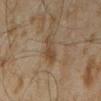Assessment: This lesion was catalogued during total-body skin photography and was not selected for biopsy. Image and clinical context: About 3.5 mm across. From the left forearm. A 15 mm close-up extracted from a 3D total-body photography capture. The tile uses cross-polarized illumination. A male subject in their mid-40s.